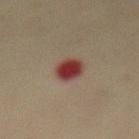Impression: This lesion was catalogued during total-body skin photography and was not selected for biopsy. Acquisition and patient details: The lesion's longest dimension is about 3.5 mm. Automated image analysis of the tile measured an area of roughly 7.5 mm², an outline eccentricity of about 0.65 (0 = round, 1 = elongated), and a shape-asymmetry score of about 0.1 (0 = symmetric). And it measured border irregularity of about 1 on a 0–10 scale, a color-variation rating of about 4/10, and a peripheral color-asymmetry measure near 1. It also reported a nevus-likeness score of about 0/100 and lesion-presence confidence of about 100/100. On the abdomen. A 15 mm close-up tile from a total-body photography series done for melanoma screening. A female subject about 80 years old.